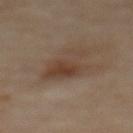Findings:
– lesion size · about 6 mm
– image-analysis metrics · a lesion–skin lightness drop of about 7; an automated nevus-likeness rating near 60 out of 100 and lesion-presence confidence of about 100/100
– patient · female, aged around 60
– anatomic site · the back
– acquisition · total-body-photography crop, ~15 mm field of view
– lighting · cross-polarized illumination